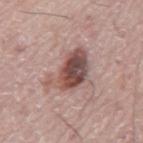Imaged during a routine full-body skin examination; the lesion was not biopsied and no histopathology is available. A male patient aged 73–77. On the mid back. Automated image analysis of the tile measured a footprint of about 14 mm² and a shape eccentricity near 0.8. A close-up tile cropped from a whole-body skin photograph, about 15 mm across. The lesion's longest dimension is about 5.5 mm.